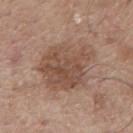Recorded during total-body skin imaging; not selected for excision or biopsy. This image is a 15 mm lesion crop taken from a total-body photograph. This is a white-light tile. The patient is a male aged around 75. About 7 mm across. The lesion is located on the back.